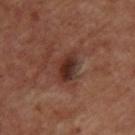Part of a total-body skin-imaging series; this lesion was reviewed on a skin check and was not flagged for biopsy. Approximately 4 mm at its widest. On the upper back. Captured under cross-polarized illumination. Cropped from a whole-body photographic skin survey; the tile spans about 15 mm. A female patient, in their mid- to late 40s.